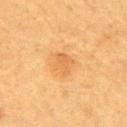Clinical impression:
The lesion was tiled from a total-body skin photograph and was not biopsied.
Image and clinical context:
Captured under cross-polarized illumination. Located on the upper back. The subject is aged 58 to 62. A 15 mm crop from a total-body photograph taken for skin-cancer surveillance. About 3 mm across. The total-body-photography lesion software estimated a color-variation rating of about 2/10 and peripheral color asymmetry of about 0.5.